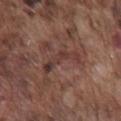Imaged during a routine full-body skin examination; the lesion was not biopsied and no histopathology is available.
The lesion is on the chest.
A male patient, aged around 75.
This image is a 15 mm lesion crop taken from a total-body photograph.
This is a white-light tile.
About 5 mm across.